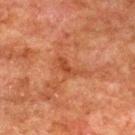Imaged during a routine full-body skin examination; the lesion was not biopsied and no histopathology is available. On the upper back. A male patient aged around 80. A 15 mm crop from a total-body photograph taken for skin-cancer surveillance. The lesion's longest dimension is about 4 mm.